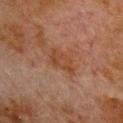Part of a total-body skin-imaging series; this lesion was reviewed on a skin check and was not flagged for biopsy.
On the chest.
Approximately 4 mm at its widest.
A close-up tile cropped from a whole-body skin photograph, about 15 mm across.
A male patient approximately 80 years of age.
Automated tile analysis of the lesion measured a lesion area of about 6 mm² and two-axis asymmetry of about 0.35. The software also gave a lesion color around L≈34 a*≈18 b*≈27 in CIELAB, about 5 CIELAB-L* units darker than the surrounding skin, and a normalized lesion–skin contrast near 7. The analysis additionally found a border-irregularity rating of about 4/10 and a color-variation rating of about 3/10. The analysis additionally found an automated nevus-likeness rating near 0 out of 100.